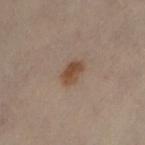{"biopsy_status": "not biopsied; imaged during a skin examination", "site": "leg", "automated_metrics": {"shape_asymmetry": 0.15, "cielab_L": 39, "cielab_a": 14, "cielab_b": 25, "border_irregularity_0_10": 1.5, "color_variation_0_10": 2.5}, "patient": {"sex": "female", "age_approx": 50}, "image": {"source": "total-body photography crop", "field_of_view_mm": 15}, "lighting": "cross-polarized", "lesion_size": {"long_diameter_mm_approx": 3.0}}